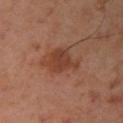| feature | finding |
|---|---|
| biopsy status | no biopsy performed (imaged during a skin exam) |
| body site | the right upper arm |
| illumination | cross-polarized |
| acquisition | total-body-photography crop, ~15 mm field of view |
| patient | female, aged approximately 45 |